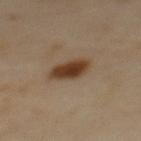| key | value |
|---|---|
| follow-up | catalogued during a skin exam; not biopsied |
| image source | 15 mm crop, total-body photography |
| lighting | cross-polarized illumination |
| diameter | ≈4 mm |
| location | the back |
| subject | male, in their mid- to late 60s |